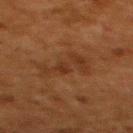Clinical impression:
This lesion was catalogued during total-body skin photography and was not selected for biopsy.
Background:
A female patient aged 48 to 52. The lesion-visualizer software estimated a color-variation rating of about 3/10 and a peripheral color-asymmetry measure near 1. It also reported lesion-presence confidence of about 100/100. Cropped from a whole-body photographic skin survey; the tile spans about 15 mm. Imaged with cross-polarized lighting. The lesion is on the upper back. The lesion's longest dimension is about 5 mm.